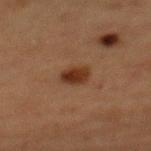Impression:
This lesion was catalogued during total-body skin photography and was not selected for biopsy.
Background:
A female subject aged 58 to 62. This is a cross-polarized tile. Measured at roughly 3 mm in maximum diameter. This image is a 15 mm lesion crop taken from a total-body photograph. Automated tile analysis of the lesion measured a lesion color around L≈27 a*≈18 b*≈26 in CIELAB, about 10 CIELAB-L* units darker than the surrounding skin, and a lesion-to-skin contrast of about 10 (normalized; higher = more distinct). It also reported radial color variation of about 1.5. From the mid back.